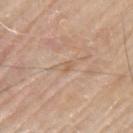The lesion was tiled from a total-body skin photograph and was not biopsied. Automated tile analysis of the lesion measured an automated nevus-likeness rating near 0 out of 100. Cropped from a whole-body photographic skin survey; the tile spans about 15 mm. The lesion is located on the upper back. About 2.5 mm across. The subject is a male aged 78–82. The tile uses white-light illumination.